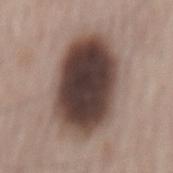<lesion>
<lesion_size>
  <long_diameter_mm_approx>9.0</long_diameter_mm_approx>
</lesion_size>
<site>mid back</site>
<image>
  <source>total-body photography crop</source>
  <field_of_view_mm>15</field_of_view_mm>
</image>
<patient>
  <sex>male</sex>
  <age_approx>65</age_approx>
</patient>
</lesion>